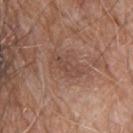notes: imaged on a skin check; not biopsied